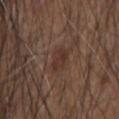Clinical impression:
The lesion was tiled from a total-body skin photograph and was not biopsied.
Image and clinical context:
Captured under white-light illumination. Located on the right forearm. This image is a 15 mm lesion crop taken from a total-body photograph. Automated image analysis of the tile measured a lesion area of about 4.5 mm² and a shape-asymmetry score of about 0.25 (0 = symmetric). The software also gave a border-irregularity rating of about 2/10 and a color-variation rating of about 2/10. It also reported a classifier nevus-likeness of about 5/100 and a detector confidence of about 100 out of 100 that the crop contains a lesion. A male subject aged approximately 50. The lesion's longest dimension is about 3 mm.